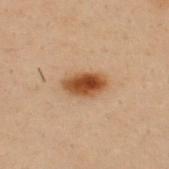Q: Was this lesion biopsied?
A: catalogued during a skin exam; not biopsied